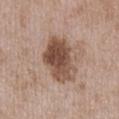The lesion was tiled from a total-body skin photograph and was not biopsied. The lesion is located on the chest. A male subject aged around 50. Cropped from a whole-body photographic skin survey; the tile spans about 15 mm. Measured at roughly 6 mm in maximum diameter.